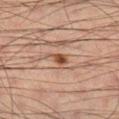Q: Was a biopsy performed?
A: no biopsy performed (imaged during a skin exam)
Q: What did automated image analysis measure?
A: a lesion area of about 3.5 mm², a shape eccentricity near 0.8, and a shape-asymmetry score of about 0.4 (0 = symmetric); a border-irregularity index near 4.5/10 and peripheral color asymmetry of about 1; a classifier nevus-likeness of about 95/100 and a lesion-detection confidence of about 100/100
Q: What are the patient's age and sex?
A: male, in their mid- to late 30s
Q: What is the imaging modality?
A: total-body-photography crop, ~15 mm field of view
Q: What is the lesion's diameter?
A: about 3 mm
Q: Lesion location?
A: the right lower leg